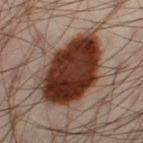notes: total-body-photography surveillance lesion; no biopsy | automated lesion analysis: a lesion area of about 37 mm², an outline eccentricity of about 0.8 (0 = round, 1 = elongated), and a shape-asymmetry score of about 0.15 (0 = symmetric) | lesion size: ≈9 mm | location: the left thigh | illumination: cross-polarized | patient: male, aged approximately 40 | image: 15 mm crop, total-body photography.